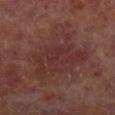Findings:
• workup · total-body-photography surveillance lesion; no biopsy
• image · ~15 mm crop, total-body skin-cancer survey
• size · ≈10 mm
• subject · male, aged 63–67
• image-analysis metrics · a border-irregularity rating of about 7.5/10, a within-lesion color-variation index near 4/10, and peripheral color asymmetry of about 1.5; a nevus-likeness score of about 0/100 and a lesion-detection confidence of about 100/100
• location · the left lower leg